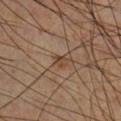Imaged during a routine full-body skin examination; the lesion was not biopsied and no histopathology is available. Captured under cross-polarized illumination. The subject is a male aged around 60. Cropped from a whole-body photographic skin survey; the tile spans about 15 mm. Approximately 1.5 mm at its widest. Automated image analysis of the tile measured a footprint of about 1.5 mm², an outline eccentricity of about 0.6 (0 = round, 1 = elongated), and two-axis asymmetry of about 0.45. It also reported an average lesion color of about L≈40 a*≈16 b*≈28 (CIELAB) and a normalized lesion–skin contrast near 7.5. It also reported a border-irregularity rating of about 4/10, internal color variation of about 0 on a 0–10 scale, and a peripheral color-asymmetry measure near 0. And it measured a lesion-detection confidence of about 100/100. The lesion is on the right lower leg.